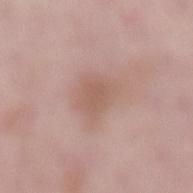biopsy status: total-body-photography surveillance lesion; no biopsy
image source: ~15 mm crop, total-body skin-cancer survey
subject: male, roughly 55 years of age
location: the lower back